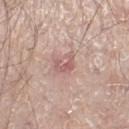Findings:
* notes — imaged on a skin check; not biopsied
* tile lighting — white-light illumination
* subject — male, aged 58 to 62
* image — total-body-photography crop, ~15 mm field of view
* lesion diameter — ~3 mm (longest diameter)
* body site — the right lower leg